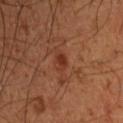Context:
The lesion is on the back. This image is a 15 mm lesion crop taken from a total-body photograph. A male subject aged approximately 50.